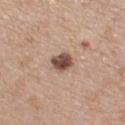Clinical summary: Imaged with white-light lighting. A female subject, aged approximately 45. Longest diameter approximately 2.5 mm. From the front of the torso. A close-up tile cropped from a whole-body skin photograph, about 15 mm across.